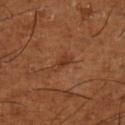site — the left lower leg; patient — male, about 65 years old; image source — 15 mm crop, total-body photography.